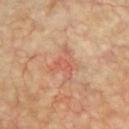The total-body-photography lesion software estimated a classifier nevus-likeness of about 0/100 and lesion-presence confidence of about 100/100. This is a cross-polarized tile. About 3.5 mm across. The patient is a male approximately 60 years of age. The lesion is located on the chest. Cropped from a whole-body photographic skin survey; the tile spans about 15 mm.